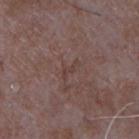Captured during whole-body skin photography for melanoma surveillance; the lesion was not biopsied.
The lesion-visualizer software estimated a normalized border contrast of about 5. The software also gave border irregularity of about 5.5 on a 0–10 scale, a color-variation rating of about 0/10, and peripheral color asymmetry of about 0. The analysis additionally found an automated nevus-likeness rating near 0 out of 100 and lesion-presence confidence of about 80/100.
Imaged with white-light lighting.
Cropped from a whole-body photographic skin survey; the tile spans about 15 mm.
On the chest.
A male patient aged around 65.